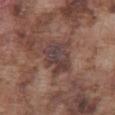Q: Was this lesion biopsied?
A: total-body-photography surveillance lesion; no biopsy
Q: Automated lesion metrics?
A: a lesion color around L≈39 a*≈17 b*≈19 in CIELAB, about 9 CIELAB-L* units darker than the surrounding skin, and a normalized border contrast of about 8.5; border irregularity of about 3.5 on a 0–10 scale and a peripheral color-asymmetry measure near 2; a classifier nevus-likeness of about 0/100
Q: What kind of image is this?
A: total-body-photography crop, ~15 mm field of view
Q: What are the patient's age and sex?
A: male, aged approximately 75
Q: Lesion location?
A: the front of the torso
Q: How was the tile lit?
A: white-light
Q: What is the lesion's diameter?
A: about 4.5 mm